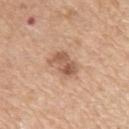• biopsy status · total-body-photography surveillance lesion; no biopsy
• lesion size · ~4 mm (longest diameter)
• location · the left upper arm
• illumination · white-light
• acquisition · 15 mm crop, total-body photography
• subject · male, aged approximately 60